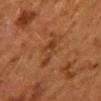The lesion was photographed on a routine skin check and not biopsied; there is no pathology result.
A 15 mm close-up tile from a total-body photography series done for melanoma screening.
The subject is a female aged approximately 50.
Captured under cross-polarized illumination.
From the mid back.
Approximately 4.5 mm at its widest.